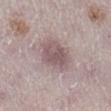The lesion was tiled from a total-body skin photograph and was not biopsied. The total-body-photography lesion software estimated peripheral color asymmetry of about 1. It also reported a nevus-likeness score of about 20/100 and a lesion-detection confidence of about 60/100. This image is a 15 mm lesion crop taken from a total-body photograph. This is a white-light tile. Located on the right lower leg. Measured at roughly 4.5 mm in maximum diameter. A female subject aged 48–52.